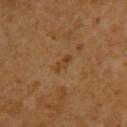A female patient aged 53 to 57.
Located on the back.
A close-up tile cropped from a whole-body skin photograph, about 15 mm across.
About 2.5 mm across.
This is a cross-polarized tile.
The total-body-photography lesion software estimated a shape eccentricity near 0.9 and two-axis asymmetry of about 0.35. It also reported a border-irregularity rating of about 3.5/10 and a color-variation rating of about 0/10.